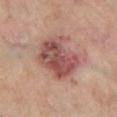Context: Located on the left lower leg. The tile uses cross-polarized illumination. The patient is a male aged 68–72. Cropped from a whole-body photographic skin survey; the tile spans about 15 mm. The total-body-photography lesion software estimated a mean CIELAB color near L≈49 a*≈23 b*≈23, roughly 12 lightness units darker than nearby skin, and a lesion-to-skin contrast of about 9 (normalized; higher = more distinct). And it measured border irregularity of about 3 on a 0–10 scale and a color-variation rating of about 7/10. The analysis additionally found a nevus-likeness score of about 20/100 and a detector confidence of about 100 out of 100 that the crop contains a lesion. Measured at roughly 5.5 mm in maximum diameter.